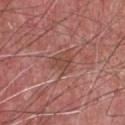Captured under white-light illumination.
A male patient, aged around 55.
The lesion's longest dimension is about 3.5 mm.
From the chest.
A 15 mm crop from a total-body photograph taken for skin-cancer surveillance.
Automated tile analysis of the lesion measured a classifier nevus-likeness of about 0/100 and a detector confidence of about 95 out of 100 that the crop contains a lesion.